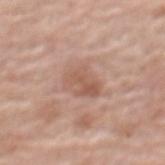The lesion was photographed on a routine skin check and not biopsied; there is no pathology result. A region of skin cropped from a whole-body photographic capture, roughly 15 mm wide. The lesion-visualizer software estimated a lesion color around L≈57 a*≈20 b*≈28 in CIELAB, about 8 CIELAB-L* units darker than the surrounding skin, and a normalized border contrast of about 6. Approximately 4 mm at its widest. From the back. This is a white-light tile. The subject is a female aged 63 to 67.